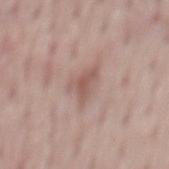Case summary:
• notes · catalogued during a skin exam; not biopsied
• image-analysis metrics · a lesion area of about 8 mm² and two-axis asymmetry of about 0.45; an average lesion color of about L≈57 a*≈18 b*≈23 (CIELAB), roughly 8 lightness units darker than nearby skin, and a lesion-to-skin contrast of about 6 (normalized; higher = more distinct); border irregularity of about 5.5 on a 0–10 scale, a within-lesion color-variation index near 2.5/10, and radial color variation of about 1; an automated nevus-likeness rating near 0 out of 100 and a detector confidence of about 100 out of 100 that the crop contains a lesion
• location · the back
• image · ~15 mm crop, total-body skin-cancer survey
• patient · male, aged approximately 55